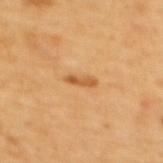Clinical impression: Imaged during a routine full-body skin examination; the lesion was not biopsied and no histopathology is available. Context: The patient is a female aged around 55. A 15 mm crop from a total-body photograph taken for skin-cancer surveillance. From the upper back.